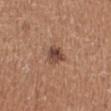{
  "biopsy_status": "not biopsied; imaged during a skin examination",
  "patient": {
    "sex": "female",
    "age_approx": 35
  },
  "image": {
    "source": "total-body photography crop",
    "field_of_view_mm": 15
  },
  "lighting": "white-light",
  "site": "right lower leg",
  "automated_metrics": {
    "border_irregularity_0_10": 2.5,
    "color_variation_0_10": 5.5,
    "peripheral_color_asymmetry": 2.0,
    "lesion_detection_confidence_0_100": 100
  },
  "lesion_size": {
    "long_diameter_mm_approx": 2.5
  }
}